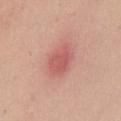Q: Was a biopsy performed?
A: total-body-photography surveillance lesion; no biopsy
Q: Lesion location?
A: the chest
Q: How large is the lesion?
A: ≈3.5 mm
Q: Who is the patient?
A: female, approximately 65 years of age
Q: How was this image acquired?
A: ~15 mm crop, total-body skin-cancer survey